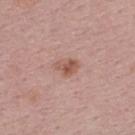biopsy_status: not biopsied; imaged during a skin examination
patient:
  sex: male
  age_approx: 30
automated_metrics:
  area_mm2_approx: 3.5
  eccentricity: 0.7
  shape_asymmetry: 0.35
  cielab_L: 54
  cielab_a: 23
  cielab_b: 27
  vs_skin_contrast_norm: 7.5
  border_irregularity_0_10: 3.5
  color_variation_0_10: 2.0
  peripheral_color_asymmetry: 1.0
  nevus_likeness_0_100: 65
  lesion_detection_confidence_0_100: 100
image:
  source: total-body photography crop
  field_of_view_mm: 15